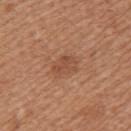| feature | finding |
|---|---|
| workup | catalogued during a skin exam; not biopsied |
| body site | the left upper arm |
| TBP lesion metrics | a lesion area of about 5 mm² and a shape eccentricity near 0.7; a mean CIELAB color near L≈49 a*≈23 b*≈32, roughly 7 lightness units darker than nearby skin, and a normalized lesion–skin contrast near 5.5; a classifier nevus-likeness of about 10/100 and a detector confidence of about 100 out of 100 that the crop contains a lesion |
| subject | female, in their mid-50s |
| acquisition | ~15 mm crop, total-body skin-cancer survey |
| diameter | ~3 mm (longest diameter) |